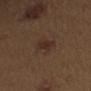Image and clinical context:
The lesion-visualizer software estimated about 6 CIELAB-L* units darker than the surrounding skin and a lesion-to-skin contrast of about 6.5 (normalized; higher = more distinct). The software also gave a nevus-likeness score of about 70/100 and lesion-presence confidence of about 100/100. This is a white-light tile. Longest diameter approximately 3 mm. A 15 mm crop from a total-body photograph taken for skin-cancer surveillance. A male patient aged approximately 70. The lesion is on the right upper arm.